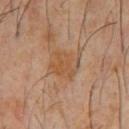<case>
<biopsy_status>not biopsied; imaged during a skin examination</biopsy_status>
<lesion_size>
  <long_diameter_mm_approx>4.0</long_diameter_mm_approx>
</lesion_size>
<site>chest</site>
<image>
  <source>total-body photography crop</source>
  <field_of_view_mm>15</field_of_view_mm>
</image>
<patient>
  <sex>male</sex>
  <age_approx>60</age_approx>
</patient>
</case>